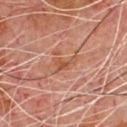Q: Is there a histopathology result?
A: imaged on a skin check; not biopsied
Q: Illumination type?
A: cross-polarized illumination
Q: How was this image acquired?
A: ~15 mm tile from a whole-body skin photo
Q: Lesion location?
A: the front of the torso
Q: What is the lesion's diameter?
A: ≈3 mm
Q: Patient demographics?
A: male, aged around 80
Q: Automated lesion metrics?
A: a lesion–skin lightness drop of about 6 and a normalized border contrast of about 6.5; lesion-presence confidence of about 100/100